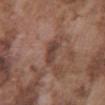Recorded during total-body skin imaging; not selected for excision or biopsy. The subject is a male in their mid- to late 70s. Located on the chest. A roughly 15 mm field-of-view crop from a total-body skin photograph.